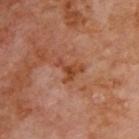workup: imaged on a skin check; not biopsied
illumination: cross-polarized illumination
lesion diameter: ≈3.5 mm
acquisition: ~15 mm tile from a whole-body skin photo
location: the back
subject: male, in their 70s